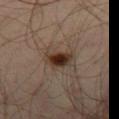{
  "lighting": "cross-polarized",
  "automated_metrics": {
    "area_mm2_approx": 7.0,
    "shape_asymmetry": 0.2,
    "border_irregularity_0_10": 2.5,
    "color_variation_0_10": 7.0,
    "peripheral_color_asymmetry": 2.0
  },
  "lesion_size": {
    "long_diameter_mm_approx": 3.5
  },
  "patient": {
    "sex": "male",
    "age_approx": 35
  },
  "site": "right thigh",
  "image": {
    "source": "total-body photography crop",
    "field_of_view_mm": 15
  }
}